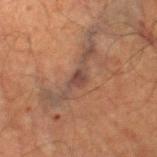Part of a total-body skin-imaging series; this lesion was reviewed on a skin check and was not flagged for biopsy.
A male patient, approximately 65 years of age.
The lesion is on the right thigh.
This image is a 15 mm lesion crop taken from a total-body photograph.